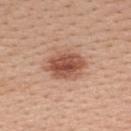Assessment:
Imaged during a routine full-body skin examination; the lesion was not biopsied and no histopathology is available.
Acquisition and patient details:
A 15 mm crop from a total-body photograph taken for skin-cancer surveillance. The recorded lesion diameter is about 5 mm. Automated tile analysis of the lesion measured an average lesion color of about L≈53 a*≈24 b*≈30 (CIELAB), roughly 14 lightness units darker than nearby skin, and a lesion-to-skin contrast of about 9 (normalized; higher = more distinct). A female patient, in their 40s. From the upper back. The tile uses white-light illumination.